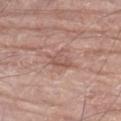acquisition: total-body-photography crop, ~15 mm field of view | lighting: white-light | site: the right lower leg | patient: male, aged 78–82.